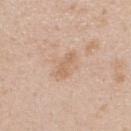notes — total-body-photography surveillance lesion; no biopsy | automated metrics — border irregularity of about 3.5 on a 0–10 scale, a color-variation rating of about 1.5/10, and a peripheral color-asymmetry measure near 0.5 | body site — the upper back | image source — 15 mm crop, total-body photography | diameter — about 3 mm | patient — male, approximately 25 years of age.